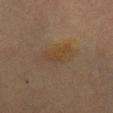  biopsy_status: not biopsied; imaged during a skin examination
  patient:
    sex: female
    age_approx: 60
  site: chest
  lesion_size:
    long_diameter_mm_approx: 3.5
  image:
    source: total-body photography crop
    field_of_view_mm: 15
  lighting: cross-polarized